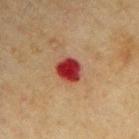The lesion was tiled from a total-body skin photograph and was not biopsied. The subject is a male aged 68 to 72. Automated tile analysis of the lesion measured an area of roughly 7 mm² and a symmetry-axis asymmetry near 0.15. The analysis additionally found an average lesion color of about L≈32 a*≈33 b*≈26 (CIELAB), a lesion–skin lightness drop of about 16, and a lesion-to-skin contrast of about 14 (normalized; higher = more distinct). It also reported a border-irregularity index near 1.5/10, internal color variation of about 3.5 on a 0–10 scale, and radial color variation of about 1. The software also gave an automated nevus-likeness rating near 0 out of 100 and a detector confidence of about 100 out of 100 that the crop contains a lesion. This image is a 15 mm lesion crop taken from a total-body photograph. From the right upper arm. Longest diameter approximately 3 mm.